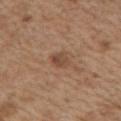  biopsy_status: not biopsied; imaged during a skin examination
  patient:
    sex: male
    age_approx: 70
  image:
    source: total-body photography crop
    field_of_view_mm: 15
  site: left upper arm